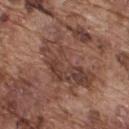size — about 7.5 mm
image — 15 mm crop, total-body photography
image-analysis metrics — a normalized lesion–skin contrast near 7; border irregularity of about 7 on a 0–10 scale, internal color variation of about 5.5 on a 0–10 scale, and peripheral color asymmetry of about 2; an automated nevus-likeness rating near 0 out of 100 and lesion-presence confidence of about 85/100
subject — male, about 75 years old
site — the upper back
illumination — white-light illumination